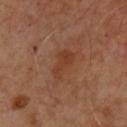The lesion was photographed on a routine skin check and not biopsied; there is no pathology result. A 15 mm crop from a total-body photograph taken for skin-cancer surveillance. The lesion is located on the chest. The patient is a male about 50 years old.